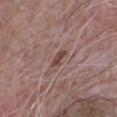| field | value |
|---|---|
| notes | imaged on a skin check; not biopsied |
| lighting | white-light illumination |
| subject | male, roughly 65 years of age |
| image | total-body-photography crop, ~15 mm field of view |
| size | ~3 mm (longest diameter) |
| body site | the front of the torso |